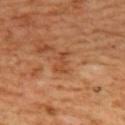| feature | finding |
|---|---|
| notes | catalogued during a skin exam; not biopsied |